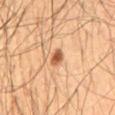Imaged during a routine full-body skin examination; the lesion was not biopsied and no histopathology is available. A region of skin cropped from a whole-body photographic capture, roughly 15 mm wide. The lesion is located on the mid back. The total-body-photography lesion software estimated a nevus-likeness score of about 100/100 and a detector confidence of about 100 out of 100 that the crop contains a lesion. The patient is a male in their mid- to late 50s.